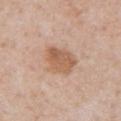biopsy_status: not biopsied; imaged during a skin examination
lighting: white-light
site: abdomen
patient:
  sex: male
  age_approx: 65
image:
  source: total-body photography crop
  field_of_view_mm: 15
automated_metrics:
  area_mm2_approx: 11.0
  shape_asymmetry: 0.25
  border_irregularity_0_10: 2.5
  color_variation_0_10: 3.5
  peripheral_color_asymmetry: 1.5